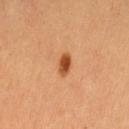<record>
<biopsy_status>not biopsied; imaged during a skin examination</biopsy_status>
<image>
  <source>total-body photography crop</source>
  <field_of_view_mm>15</field_of_view_mm>
</image>
<site>left thigh</site>
<patient>
  <age_approx>70</age_approx>
</patient>
<lesion_size>
  <long_diameter_mm_approx>2.5</long_diameter_mm_approx>
</lesion_size>
</record>